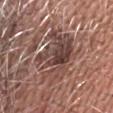Acquisition and patient details: A male subject, roughly 75 years of age. Imaged with white-light lighting. Cropped from a total-body skin-imaging series; the visible field is about 15 mm. Located on the head or neck. The lesion's longest dimension is about 7.5 mm. The lesion-visualizer software estimated a footprint of about 25 mm², an eccentricity of roughly 0.8, and a symmetry-axis asymmetry near 0.35. The software also gave a lesion color around L≈44 a*≈19 b*≈22 in CIELAB and roughly 10 lightness units darker than nearby skin. It also reported a nevus-likeness score of about 0/100.